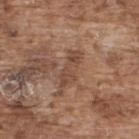{"biopsy_status": "not biopsied; imaged during a skin examination", "automated_metrics": {"area_mm2_approx": 5.5, "shape_asymmetry": 0.4, "nevus_likeness_0_100": 0}, "image": {"source": "total-body photography crop", "field_of_view_mm": 15}, "lesion_size": {"long_diameter_mm_approx": 4.5}, "patient": {"sex": "male", "age_approx": 75}, "site": "upper back"}